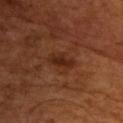Impression:
Recorded during total-body skin imaging; not selected for excision or biopsy.
Acquisition and patient details:
Approximately 3.5 mm at its widest. Automated image analysis of the tile measured a footprint of about 5 mm², a shape eccentricity near 0.9, and a shape-asymmetry score of about 0.25 (0 = symmetric). And it measured a mean CIELAB color near L≈24 a*≈21 b*≈27, roughly 7 lightness units darker than nearby skin, and a lesion-to-skin contrast of about 7.5 (normalized; higher = more distinct). A male patient, aged approximately 55. On the chest. Cropped from a total-body skin-imaging series; the visible field is about 15 mm. The tile uses cross-polarized illumination.